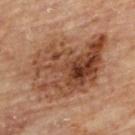Impression: The lesion was tiled from a total-body skin photograph and was not biopsied. Context: Captured under cross-polarized illumination. Automated image analysis of the tile measured an eccentricity of roughly 0.75 and a symmetry-axis asymmetry near 0.25. And it measured a border-irregularity index near 3.5/10, a within-lesion color-variation index near 9.5/10, and a peripheral color-asymmetry measure near 4. A male subject about 85 years old or older. The lesion's longest dimension is about 10 mm. A 15 mm close-up extracted from a 3D total-body photography capture. The lesion is located on the back.